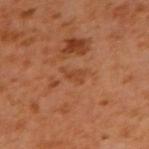notes = catalogued during a skin exam; not biopsied | tile lighting = cross-polarized illumination | size = ≈3 mm | anatomic site = the right upper arm | patient = male, approximately 60 years of age | imaging modality = 15 mm crop, total-body photography.